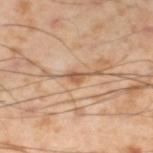Case summary:
– workup: no biopsy performed (imaged during a skin exam)
– patient: male, in their mid-50s
– location: the left lower leg
– illumination: cross-polarized
– image source: ~15 mm crop, total-body skin-cancer survey